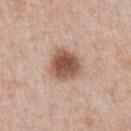lesion diameter = ~4 mm (longest diameter); tile lighting = white-light; patient = male, roughly 60 years of age; imaging modality = total-body-photography crop, ~15 mm field of view; body site = the abdomen.